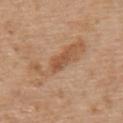biopsy status: catalogued during a skin exam; not biopsied | patient: male, aged around 70 | lighting: white-light | imaging modality: ~15 mm crop, total-body skin-cancer survey | location: the mid back | image-analysis metrics: an average lesion color of about L≈51 a*≈23 b*≈35 (CIELAB), a lesion–skin lightness drop of about 10, and a normalized lesion–skin contrast near 7; border irregularity of about 4 on a 0–10 scale, internal color variation of about 0 on a 0–10 scale, and peripheral color asymmetry of about 0; a classifier nevus-likeness of about 0/100 | size: about 3 mm.